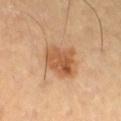The lesion was photographed on a routine skin check and not biopsied; there is no pathology result. From the right thigh. Imaged with cross-polarized lighting. The patient is aged around 65. A lesion tile, about 15 mm wide, cut from a 3D total-body photograph.